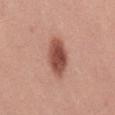From the mid back. The patient is a female about 25 years old. This image is a 15 mm lesion crop taken from a total-body photograph.